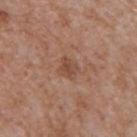Clinical impression: The lesion was tiled from a total-body skin photograph and was not biopsied. Background: The tile uses white-light illumination. A lesion tile, about 15 mm wide, cut from a 3D total-body photograph. A male patient aged 63–67. The lesion's longest dimension is about 2.5 mm. The lesion is located on the mid back.